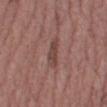The lesion was photographed on a routine skin check and not biopsied; there is no pathology result. A female patient aged approximately 40. Located on the leg. A 15 mm close-up tile from a total-body photography series done for melanoma screening.